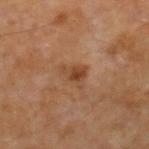This lesion was catalogued during total-body skin photography and was not selected for biopsy.
Located on the right lower leg.
A 15 mm crop from a total-body photograph taken for skin-cancer surveillance.
A male subject about 60 years old.
Automated tile analysis of the lesion measured an average lesion color of about L≈41 a*≈20 b*≈31 (CIELAB). It also reported a border-irregularity rating of about 4.5/10, a within-lesion color-variation index near 3.5/10, and a peripheral color-asymmetry measure near 1.5. The analysis additionally found a nevus-likeness score of about 20/100 and lesion-presence confidence of about 100/100.
The lesion's longest dimension is about 3.5 mm.